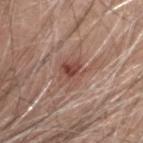Clinical impression:
The lesion was photographed on a routine skin check and not biopsied; there is no pathology result.
Image and clinical context:
Measured at roughly 3.5 mm in maximum diameter. A region of skin cropped from a whole-body photographic capture, roughly 15 mm wide. Imaged with white-light lighting. A male patient roughly 80 years of age. On the head or neck. The total-body-photography lesion software estimated a mean CIELAB color near L≈48 a*≈22 b*≈25, a lesion–skin lightness drop of about 10, and a normalized border contrast of about 7.